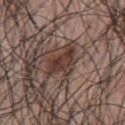– automated metrics: an area of roughly 8 mm², an eccentricity of roughly 0.8, and a shape-asymmetry score of about 0.45 (0 = symmetric); a lesion color around L≈36 a*≈18 b*≈22 in CIELAB and a lesion-to-skin contrast of about 9.5 (normalized; higher = more distinct); border irregularity of about 5 on a 0–10 scale, a within-lesion color-variation index near 4.5/10, and a peripheral color-asymmetry measure near 1.5; an automated nevus-likeness rating near 95 out of 100
– lesion size: ~4.5 mm (longest diameter)
– patient: male, aged 68 to 72
– illumination: white-light illumination
– anatomic site: the chest
– image source: ~15 mm tile from a whole-body skin photo